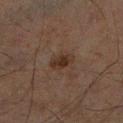<tbp_lesion>
  <biopsy_status>not biopsied; imaged during a skin examination</biopsy_status>
  <patient>
    <sex>male</sex>
    <age_approx>65</age_approx>
  </patient>
  <site>leg</site>
  <image>
    <source>total-body photography crop</source>
    <field_of_view_mm>15</field_of_view_mm>
  </image>
</tbp_lesion>